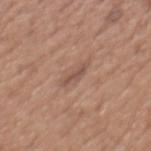workup = catalogued during a skin exam; not biopsied | site = the back | imaging modality = ~15 mm tile from a whole-body skin photo | patient = male, aged around 65.